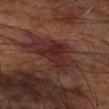No biopsy was performed on this lesion — it was imaged during a full skin examination and was not determined to be concerning. Automated image analysis of the tile measured a lesion area of about 18 mm², an outline eccentricity of about 0.8 (0 = round, 1 = elongated), and a symmetry-axis asymmetry near 0.4. It also reported a lesion–skin lightness drop of about 7 and a normalized lesion–skin contrast near 8.5. And it measured a border-irregularity rating of about 5/10, a within-lesion color-variation index near 5/10, and radial color variation of about 1.5. And it measured a nevus-likeness score of about 0/100 and lesion-presence confidence of about 85/100. The lesion is located on the arm. Cropped from a total-body skin-imaging series; the visible field is about 15 mm. Captured under cross-polarized illumination. A male patient roughly 60 years of age.